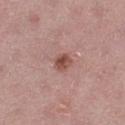notes=catalogued during a skin exam; not biopsied | patient=female, aged approximately 45 | tile lighting=white-light illumination | body site=the left thigh | image=~15 mm tile from a whole-body skin photo | size=about 2.5 mm.